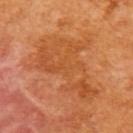| key | value |
|---|---|
| follow-up | no biopsy performed (imaged during a skin exam) |
| automated lesion analysis | a lesion area of about 34 mm², an outline eccentricity of about 0.8 (0 = round, 1 = elongated), and two-axis asymmetry of about 0.45; a border-irregularity index near 7/10 and a peripheral color-asymmetry measure near 1.5; an automated nevus-likeness rating near 0 out of 100 and lesion-presence confidence of about 100/100 |
| patient | female, aged 53 to 57 |
| acquisition | 15 mm crop, total-body photography |
| site | the upper back |
| diameter | ~9 mm (longest diameter) |